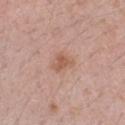• workup · total-body-photography surveillance lesion; no biopsy
• lighting · white-light
• image · ~15 mm crop, total-body skin-cancer survey
• lesion diameter · about 3 mm
• subject · female, aged 33 to 37
• anatomic site · the left forearm
• automated lesion analysis · an area of roughly 5 mm², a shape eccentricity near 0.55, and a symmetry-axis asymmetry near 0.3; a border-irregularity index near 3/10 and a color-variation rating of about 2.5/10; an automated nevus-likeness rating near 20 out of 100